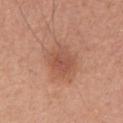lighting: white-light | subject: female, aged approximately 40 | size: ≈3.5 mm | anatomic site: the right upper arm | image: 15 mm crop, total-body photography | automated metrics: a mean CIELAB color near L≈53 a*≈24 b*≈31, roughly 8 lightness units darker than nearby skin, and a normalized border contrast of about 6.